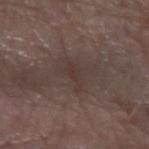Background:
A lesion tile, about 15 mm wide, cut from a 3D total-body photograph. The tile uses white-light illumination. Longest diameter approximately 3.5 mm. From the arm. A female patient in their 50s.